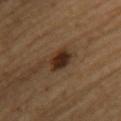Assessment: Part of a total-body skin-imaging series; this lesion was reviewed on a skin check and was not flagged for biopsy. Acquisition and patient details: A lesion tile, about 15 mm wide, cut from a 3D total-body photograph. On the back. The subject is a female aged 38 to 42.